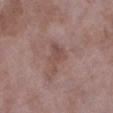The lesion was tiled from a total-body skin photograph and was not biopsied.
The patient is a female aged 68 to 72.
A 15 mm close-up extracted from a 3D total-body photography capture.
On the left lower leg.